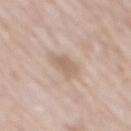imaging modality: 15 mm crop, total-body photography | body site: the mid back | image-analysis metrics: a lesion-to-skin contrast of about 6 (normalized; higher = more distinct); a border-irregularity rating of about 2.5/10, internal color variation of about 2 on a 0–10 scale, and a peripheral color-asymmetry measure near 0.5; a nevus-likeness score of about 5/100 | patient: male, approximately 80 years of age.